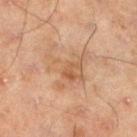Captured during whole-body skin photography for melanoma surveillance; the lesion was not biopsied.
A 15 mm close-up tile from a total-body photography series done for melanoma screening.
A male patient about 45 years old.
Imaged with cross-polarized lighting.
From the left lower leg.
About 5.5 mm across.